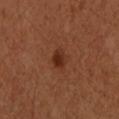No biopsy was performed on this lesion — it was imaged during a full skin examination and was not determined to be concerning.
Automated tile analysis of the lesion measured a shape eccentricity near 0.65. The analysis additionally found a mean CIELAB color near L≈29 a*≈24 b*≈30 and a normalized border contrast of about 8.5. And it measured border irregularity of about 2 on a 0–10 scale, a within-lesion color-variation index near 2.5/10, and peripheral color asymmetry of about 1.
A female subject, in their mid- to late 40s.
Longest diameter approximately 2 mm.
A 15 mm close-up extracted from a 3D total-body photography capture.
The tile uses cross-polarized illumination.
The lesion is on the right forearm.